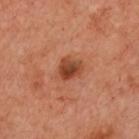Assessment:
Recorded during total-body skin imaging; not selected for excision or biopsy.
Background:
From the front of the torso. A roughly 15 mm field-of-view crop from a total-body skin photograph. The lesion's longest dimension is about 3 mm. This is a cross-polarized tile. A female patient about 55 years old.